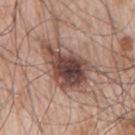patient = male, roughly 65 years of age
site = the right upper arm
automated lesion analysis = border irregularity of about 4.5 on a 0–10 scale, internal color variation of about 9 on a 0–10 scale, and peripheral color asymmetry of about 2.5; a classifier nevus-likeness of about 90/100 and lesion-presence confidence of about 100/100
lesion diameter = ≈7.5 mm
acquisition = 15 mm crop, total-body photography